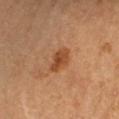Cropped from a total-body skin-imaging series; the visible field is about 15 mm.
This is a cross-polarized tile.
A female subject, in their mid- to late 60s.
The lesion is located on the arm.
Automated tile analysis of the lesion measured a mean CIELAB color near L≈46 a*≈24 b*≈37, about 10 CIELAB-L* units darker than the surrounding skin, and a lesion-to-skin contrast of about 8 (normalized; higher = more distinct). It also reported border irregularity of about 2 on a 0–10 scale, internal color variation of about 3 on a 0–10 scale, and peripheral color asymmetry of about 1. It also reported an automated nevus-likeness rating near 85 out of 100.
About 3.5 mm across.